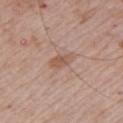Recorded during total-body skin imaging; not selected for excision or biopsy.
From the left upper arm.
Imaged with white-light lighting.
A male subject, in their mid- to late 60s.
A 15 mm close-up extracted from a 3D total-body photography capture.
An algorithmic analysis of the crop reported a shape eccentricity near 0.85 and a shape-asymmetry score of about 0.2 (0 = symmetric). The analysis additionally found an automated nevus-likeness rating near 0 out of 100 and lesion-presence confidence of about 100/100.
The lesion's longest dimension is about 3 mm.